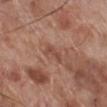biopsy status: catalogued during a skin exam; not biopsied
automated lesion analysis: radial color variation of about 0
site: the right lower leg
size: ~2.5 mm (longest diameter)
image source: 15 mm crop, total-body photography
tile lighting: white-light illumination
patient: male, roughly 70 years of age